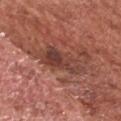{"biopsy_status": "not biopsied; imaged during a skin examination", "automated_metrics": {"area_mm2_approx": 10.0, "eccentricity": 0.95, "shape_asymmetry": 0.4, "cielab_L": 41, "cielab_a": 23, "cielab_b": 25, "vs_skin_darker_L": 10.0, "nevus_likeness_0_100": 35}, "image": {"source": "total-body photography crop", "field_of_view_mm": 15}, "lesion_size": {"long_diameter_mm_approx": 6.0}, "site": "head or neck", "patient": {"sex": "male", "age_approx": 65}, "lighting": "white-light"}